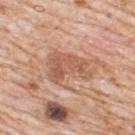biopsy status: catalogued during a skin exam; not biopsied | lesion size: ~5 mm (longest diameter) | TBP lesion metrics: an outline eccentricity of about 0.8 (0 = round, 1 = elongated) and two-axis asymmetry of about 0.5; a lesion color around L≈57 a*≈22 b*≈31 in CIELAB, roughly 10 lightness units darker than nearby skin, and a lesion-to-skin contrast of about 6.5 (normalized; higher = more distinct); border irregularity of about 6.5 on a 0–10 scale, a within-lesion color-variation index near 3.5/10, and a peripheral color-asymmetry measure near 1; an automated nevus-likeness rating near 0 out of 100 | tile lighting: white-light | patient: male, aged around 80 | image source: ~15 mm crop, total-body skin-cancer survey | anatomic site: the back.